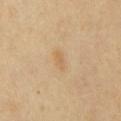On the front of the torso. About 2.5 mm across. Imaged with cross-polarized lighting. A close-up tile cropped from a whole-body skin photograph, about 15 mm across. A male subject, aged approximately 65. Automated image analysis of the tile measured an area of roughly 3 mm², an outline eccentricity of about 0.85 (0 = round, 1 = elongated), and a shape-asymmetry score of about 0.3 (0 = symmetric). And it measured an automated nevus-likeness rating near 5 out of 100.